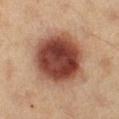This lesion was catalogued during total-body skin photography and was not selected for biopsy. The tile uses cross-polarized illumination. A roughly 15 mm field-of-view crop from a total-body skin photograph. A female subject aged around 40. The lesion is located on the left lower leg. The total-body-photography lesion software estimated a border-irregularity index near 1.5/10, internal color variation of about 7 on a 0–10 scale, and peripheral color asymmetry of about 1.5. The software also gave an automated nevus-likeness rating near 100 out of 100 and a detector confidence of about 100 out of 100 that the crop contains a lesion. Longest diameter approximately 7 mm.